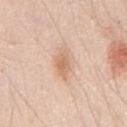{"biopsy_status": "not biopsied; imaged during a skin examination", "automated_metrics": {"border_irregularity_0_10": 3.0, "color_variation_0_10": 4.0, "peripheral_color_asymmetry": 1.0}, "patient": {"sex": "male", "age_approx": 45}, "image": {"source": "total-body photography crop", "field_of_view_mm": 15}, "lighting": "white-light", "site": "chest"}